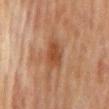<case>
<biopsy_status>not biopsied; imaged during a skin examination</biopsy_status>
</case>